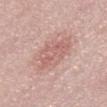Notes:
- biopsy status: imaged on a skin check; not biopsied
- acquisition: ~15 mm tile from a whole-body skin photo
- patient: male, aged approximately 55
- location: the abdomen